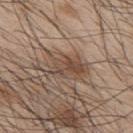Imaged during a routine full-body skin examination; the lesion was not biopsied and no histopathology is available. This is a white-light tile. Automated image analysis of the tile measured a border-irregularity index near 4.5/10 and internal color variation of about 5.5 on a 0–10 scale. The lesion is on the back. The lesion's longest dimension is about 5 mm. A male patient aged 43–47. A region of skin cropped from a whole-body photographic capture, roughly 15 mm wide.